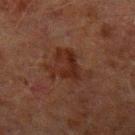Case summary:
- follow-up: catalogued during a skin exam; not biopsied
- anatomic site: the left upper arm
- patient: male, aged 58 to 62
- automated metrics: a footprint of about 13 mm², a shape eccentricity near 0.7, and two-axis asymmetry of about 0.55; a lesion color around L≈21 a*≈17 b*≈21 in CIELAB and a normalized lesion–skin contrast near 7; a border-irregularity index near 7.5/10, a color-variation rating of about 3.5/10, and a peripheral color-asymmetry measure near 1.5; a nevus-likeness score of about 40/100
- image: 15 mm crop, total-body photography
- size: about 5 mm